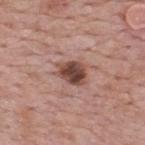Case summary:
– image source · total-body-photography crop, ~15 mm field of view
– image-analysis metrics · a lesion area of about 7.5 mm² and an eccentricity of roughly 0.7; peripheral color asymmetry of about 1.5
– lighting · white-light
– lesion size · about 3.5 mm
– site · the upper back
– patient · male, aged 53–57